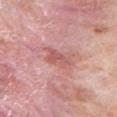Located on the arm. A male patient, in their 80s. A lesion tile, about 15 mm wide, cut from a 3D total-body photograph.